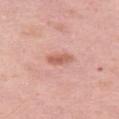{
  "biopsy_status": "not biopsied; imaged during a skin examination",
  "lighting": "white-light",
  "site": "left thigh",
  "automated_metrics": {
    "area_mm2_approx": 4.0,
    "eccentricity": 0.9,
    "shape_asymmetry": 0.25,
    "cielab_L": 60,
    "cielab_a": 26,
    "cielab_b": 30,
    "vs_skin_contrast_norm": 7.0,
    "border_irregularity_0_10": 2.5,
    "color_variation_0_10": 3.0,
    "peripheral_color_asymmetry": 1.0,
    "lesion_detection_confidence_0_100": 100
  },
  "patient": {
    "sex": "female",
    "age_approx": 40
  },
  "image": {
    "source": "total-body photography crop",
    "field_of_view_mm": 15
  },
  "lesion_size": {
    "long_diameter_mm_approx": 3.0
  }
}